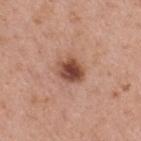biopsy status: imaged on a skin check; not biopsied | image: ~15 mm tile from a whole-body skin photo | anatomic site: the mid back | patient: male, about 40 years old.